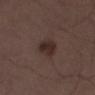Impression:
The lesion was tiled from a total-body skin photograph and was not biopsied.
Context:
The patient is a male in their 50s. Longest diameter approximately 3 mm. Cropped from a whole-body photographic skin survey; the tile spans about 15 mm. Located on the left thigh.